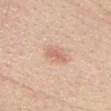Assessment: Part of a total-body skin-imaging series; this lesion was reviewed on a skin check and was not flagged for biopsy. Background: The recorded lesion diameter is about 2.5 mm. A roughly 15 mm field-of-view crop from a total-body skin photograph. Captured under white-light illumination. Automated tile analysis of the lesion measured roughly 9 lightness units darker than nearby skin and a lesion-to-skin contrast of about 5.5 (normalized; higher = more distinct). The software also gave a border-irregularity index near 3/10 and a within-lesion color-variation index near 1/10. From the head or neck. The subject is a female approximately 45 years of age.